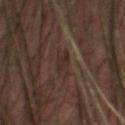biopsy_status: not biopsied; imaged during a skin examination
lesion_size:
  long_diameter_mm_approx: 2.5
site: head or neck
image:
  source: total-body photography crop
  field_of_view_mm: 15
patient:
  sex: male
  age_approx: 60
automated_metrics:
  color_variation_0_10: 1.5
  peripheral_color_asymmetry: 0.5